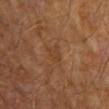Q: Was this lesion biopsied?
A: total-body-photography surveillance lesion; no biopsy
Q: What are the patient's age and sex?
A: male, aged 68 to 72
Q: Illumination type?
A: cross-polarized illumination
Q: How was this image acquired?
A: 15 mm crop, total-body photography
Q: What is the anatomic site?
A: the left upper arm
Q: Automated lesion metrics?
A: an eccentricity of roughly 0.8; a border-irregularity index near 4/10 and internal color variation of about 1.5 on a 0–10 scale